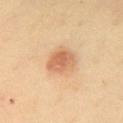This lesion was catalogued during total-body skin photography and was not selected for biopsy. The tile uses cross-polarized illumination. A lesion tile, about 15 mm wide, cut from a 3D total-body photograph. Located on the chest. Measured at roughly 3.5 mm in maximum diameter. An algorithmic analysis of the crop reported a lesion area of about 9 mm². The analysis additionally found a mean CIELAB color near L≈66 a*≈23 b*≈38 and roughly 12 lightness units darker than nearby skin. The software also gave a nevus-likeness score of about 100/100. A female patient roughly 40 years of age.